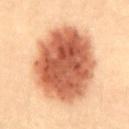Assessment:
No biopsy was performed on this lesion — it was imaged during a full skin examination and was not determined to be concerning.
Acquisition and patient details:
Captured under cross-polarized illumination. Located on the front of the torso. The total-body-photography lesion software estimated a border-irregularity rating of about 1.5/10, a color-variation rating of about 6/10, and a peripheral color-asymmetry measure near 2. A 15 mm crop from a total-body photograph taken for skin-cancer surveillance. A female subject about 20 years old.